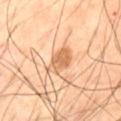A lesion tile, about 15 mm wide, cut from a 3D total-body photograph.
From the front of the torso.
Automated image analysis of the tile measured a footprint of about 6.5 mm², a shape eccentricity near 0.8, and a symmetry-axis asymmetry near 0.25. The software also gave a lesion color around L≈61 a*≈21 b*≈37 in CIELAB, a lesion–skin lightness drop of about 11, and a lesion-to-skin contrast of about 7.5 (normalized; higher = more distinct). And it measured a border-irregularity index near 2.5/10, internal color variation of about 4 on a 0–10 scale, and a peripheral color-asymmetry measure near 1.5.
A male patient aged approximately 55.